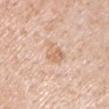illumination: white-light
site: the front of the torso
imaging modality: total-body-photography crop, ~15 mm field of view
size: ≈2.5 mm
subject: male, roughly 70 years of age